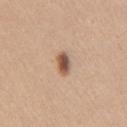follow-up: no biopsy performed (imaged during a skin exam) | illumination: white-light | patient: female, approximately 25 years of age | image: total-body-photography crop, ~15 mm field of view | lesion diameter: about 3 mm | location: the right upper arm.